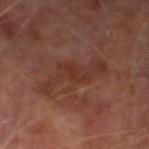Imaged during a routine full-body skin examination; the lesion was not biopsied and no histopathology is available.
This is a cross-polarized tile.
The total-body-photography lesion software estimated an automated nevus-likeness rating near 0 out of 100.
A roughly 15 mm field-of-view crop from a total-body skin photograph.
The lesion is located on the left lower leg.
Measured at roughly 7 mm in maximum diameter.
A male subject in their mid-60s.